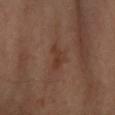Impression:
Part of a total-body skin-imaging series; this lesion was reviewed on a skin check and was not flagged for biopsy.
Context:
The subject is a female roughly 60 years of age. The lesion is located on the right forearm. A 15 mm crop from a total-body photograph taken for skin-cancer surveillance.